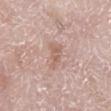This lesion was catalogued during total-body skin photography and was not selected for biopsy.
An algorithmic analysis of the crop reported a shape-asymmetry score of about 0.5 (0 = symmetric). The analysis additionally found border irregularity of about 5 on a 0–10 scale, a color-variation rating of about 2/10, and peripheral color asymmetry of about 0.5.
Captured under white-light illumination.
The lesion is located on the right lower leg.
The patient is a male aged around 80.
The recorded lesion diameter is about 3.5 mm.
A close-up tile cropped from a whole-body skin photograph, about 15 mm across.